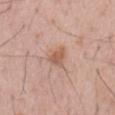Captured during whole-body skin photography for melanoma surveillance; the lesion was not biopsied. The lesion's longest dimension is about 2.5 mm. On the mid back. This image is a 15 mm lesion crop taken from a total-body photograph. A male patient approximately 55 years of age. The total-body-photography lesion software estimated an area of roughly 4 mm² and a shape eccentricity near 0.55. The software also gave a mean CIELAB color near L≈58 a*≈21 b*≈29, a lesion–skin lightness drop of about 9, and a normalized lesion–skin contrast near 7. Captured under white-light illumination.